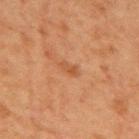Impression: Part of a total-body skin-imaging series; this lesion was reviewed on a skin check and was not flagged for biopsy. Background: A region of skin cropped from a whole-body photographic capture, roughly 15 mm wide. A female patient aged 38 to 42. The lesion is on the mid back.